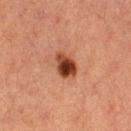Impression: The lesion was tiled from a total-body skin photograph and was not biopsied. Context: Automated image analysis of the tile measured a lesion area of about 6.5 mm², a shape eccentricity near 0.65, and a shape-asymmetry score of about 0.2 (0 = symmetric). The software also gave about 15 CIELAB-L* units darker than the surrounding skin. The patient is a female roughly 40 years of age. The lesion's longest dimension is about 3 mm. From the right thigh. A 15 mm close-up tile from a total-body photography series done for melanoma screening.